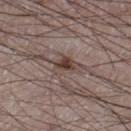Assessment: Captured during whole-body skin photography for melanoma surveillance; the lesion was not biopsied. Clinical summary: The patient is a male approximately 75 years of age. Imaged with white-light lighting. The lesion is on the right thigh. A 15 mm crop from a total-body photograph taken for skin-cancer surveillance. Approximately 4 mm at its widest. The total-body-photography lesion software estimated a within-lesion color-variation index near 6/10 and radial color variation of about 1.5. The analysis additionally found a nevus-likeness score of about 95/100 and a lesion-detection confidence of about 100/100.